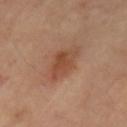Imaged during a routine full-body skin examination; the lesion was not biopsied and no histopathology is available. About 4 mm across. This image is a 15 mm lesion crop taken from a total-body photograph. An algorithmic analysis of the crop reported an outline eccentricity of about 0.7 (0 = round, 1 = elongated). And it measured a mean CIELAB color near L≈48 a*≈23 b*≈32, roughly 9 lightness units darker than nearby skin, and a normalized border contrast of about 7. The analysis additionally found a border-irregularity rating of about 3/10, internal color variation of about 3.5 on a 0–10 scale, and peripheral color asymmetry of about 1. The software also gave a nevus-likeness score of about 95/100 and a detector confidence of about 100 out of 100 that the crop contains a lesion. Imaged with cross-polarized lighting. On the left upper arm.